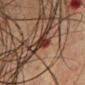notes = catalogued during a skin exam; not biopsied
illumination = cross-polarized
image = total-body-photography crop, ~15 mm field of view
size = ~2.5 mm (longest diameter)
location = the chest
subject = male, in their mid-60s